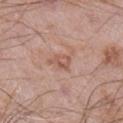| key | value |
|---|---|
| biopsy status | imaged on a skin check; not biopsied |
| size | about 2.5 mm |
| tile lighting | white-light illumination |
| body site | the right lower leg |
| subject | male, approximately 60 years of age |
| image | 15 mm crop, total-body photography |